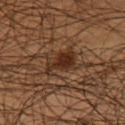From the right thigh.
A male patient, aged approximately 45.
A 15 mm close-up extracted from a 3D total-body photography capture.
Measured at roughly 4 mm in maximum diameter.
Automated tile analysis of the lesion measured an area of roughly 7 mm², an eccentricity of roughly 0.8, and a shape-asymmetry score of about 0.45 (0 = symmetric). The software also gave roughly 10 lightness units darker than nearby skin and a normalized border contrast of about 9.5. The analysis additionally found internal color variation of about 2.5 on a 0–10 scale and radial color variation of about 0.5. And it measured an automated nevus-likeness rating near 80 out of 100 and a lesion-detection confidence of about 95/100.
Imaged with cross-polarized lighting.